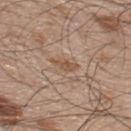{
  "biopsy_status": "not biopsied; imaged during a skin examination",
  "patient": {
    "sex": "male",
    "age_approx": 50
  },
  "lighting": "white-light",
  "image": {
    "source": "total-body photography crop",
    "field_of_view_mm": 15
  },
  "site": "back"
}